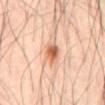Clinical impression: Recorded during total-body skin imaging; not selected for excision or biopsy. Clinical summary: This image is a 15 mm lesion crop taken from a total-body photograph. A male patient aged approximately 65. The tile uses cross-polarized illumination. The total-body-photography lesion software estimated a lesion area of about 5 mm² and an outline eccentricity of about 0.7 (0 = round, 1 = elongated). It also reported an automated nevus-likeness rating near 95 out of 100 and a lesion-detection confidence of about 100/100. The lesion is located on the abdomen.